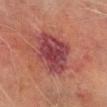Recorded during total-body skin imaging; not selected for excision or biopsy.
Automated image analysis of the tile measured an area of roughly 19 mm² and a shape eccentricity near 0.45. It also reported a mean CIELAB color near L≈34 a*≈26 b*≈18, about 10 CIELAB-L* units darker than the surrounding skin, and a normalized border contrast of about 10.5.
A male patient, roughly 70 years of age.
The lesion is located on the left lower leg.
A close-up tile cropped from a whole-body skin photograph, about 15 mm across.
About 5 mm across.